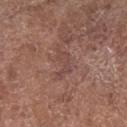Findings:
* biopsy status · no biopsy performed (imaged during a skin exam)
* TBP lesion metrics · a lesion color around L≈45 a*≈19 b*≈23 in CIELAB, about 6 CIELAB-L* units darker than the surrounding skin, and a normalized border contrast of about 5; a border-irregularity index near 7/10, internal color variation of about 1 on a 0–10 scale, and a peripheral color-asymmetry measure near 0.5; a lesion-detection confidence of about 65/100
* subject · female, approximately 80 years of age
* image source · ~15 mm tile from a whole-body skin photo
* anatomic site · the right forearm
* lesion size · about 3.5 mm
* illumination · white-light